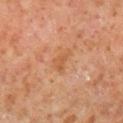Recorded during total-body skin imaging; not selected for excision or biopsy. Measured at roughly 3 mm in maximum diameter. From the right lower leg. Automated tile analysis of the lesion measured an area of roughly 5 mm², an eccentricity of roughly 0.75, and a symmetry-axis asymmetry near 0.5. The software also gave a lesion color around L≈53 a*≈23 b*≈36 in CIELAB, a lesion–skin lightness drop of about 6, and a normalized lesion–skin contrast near 5.5. The analysis additionally found a border-irregularity rating of about 4.5/10, internal color variation of about 1.5 on a 0–10 scale, and peripheral color asymmetry of about 0.5. The software also gave a classifier nevus-likeness of about 0/100. A 15 mm close-up extracted from a 3D total-body photography capture. The subject is a male about 60 years old. Captured under cross-polarized illumination.